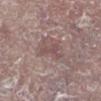Q: Is there a histopathology result?
A: imaged on a skin check; not biopsied
Q: What are the patient's age and sex?
A: male, roughly 65 years of age
Q: What lighting was used for the tile?
A: white-light
Q: How was this image acquired?
A: total-body-photography crop, ~15 mm field of view
Q: Lesion location?
A: the right lower leg